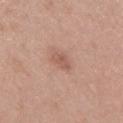Q: Was this lesion biopsied?
A: no biopsy performed (imaged during a skin exam)
Q: Illumination type?
A: white-light
Q: Where on the body is the lesion?
A: the mid back
Q: How was this image acquired?
A: ~15 mm tile from a whole-body skin photo
Q: What are the patient's age and sex?
A: female, approximately 40 years of age